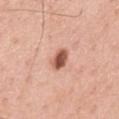Notes:
- workup · catalogued during a skin exam; not biopsied
- lesion diameter · about 3 mm
- patient · male, aged 58–62
- anatomic site · the mid back
- tile lighting · white-light
- acquisition · total-body-photography crop, ~15 mm field of view
- TBP lesion metrics · a footprint of about 5 mm², an eccentricity of roughly 0.75, and a symmetry-axis asymmetry near 0.2; an average lesion color of about L≈56 a*≈25 b*≈30 (CIELAB) and roughly 17 lightness units darker than nearby skin